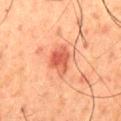biopsy status = no biopsy performed (imaged during a skin exam) | imaging modality = total-body-photography crop, ~15 mm field of view | diameter = about 3.5 mm | subject = male, aged around 60 | anatomic site = the mid back | illumination = cross-polarized.